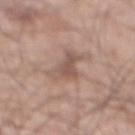notes: imaged on a skin check; not biopsied | image source: 15 mm crop, total-body photography | patient: male, aged 68 to 72 | site: the front of the torso.